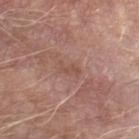Part of a total-body skin-imaging series; this lesion was reviewed on a skin check and was not flagged for biopsy.
The patient is a male about 65 years old.
On the left forearm.
A 15 mm close-up extracted from a 3D total-body photography capture.
Approximately 2.5 mm at its widest.